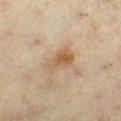Q: Is there a histopathology result?
A: no biopsy performed (imaged during a skin exam)